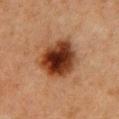Clinical impression: This lesion was catalogued during total-body skin photography and was not selected for biopsy. Background: This is a cross-polarized tile. The lesion-visualizer software estimated a footprint of about 19 mm², a shape eccentricity near 0.45, and two-axis asymmetry of about 0.15. And it measured a lesion color around L≈31 a*≈22 b*≈29 in CIELAB. And it measured border irregularity of about 1.5 on a 0–10 scale and a peripheral color-asymmetry measure near 2. It also reported an automated nevus-likeness rating near 100 out of 100 and lesion-presence confidence of about 100/100. Cropped from a whole-body photographic skin survey; the tile spans about 15 mm. On the chest. Measured at roughly 5 mm in maximum diameter. The subject is a female approximately 40 years of age.